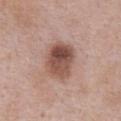<record>
  <biopsy_status>not biopsied; imaged during a skin examination</biopsy_status>
  <site>chest</site>
  <patient>
    <sex>male</sex>
    <age_approx>55</age_approx>
  </patient>
  <image>
    <source>total-body photography crop</source>
    <field_of_view_mm>15</field_of_view_mm>
  </image>
</record>